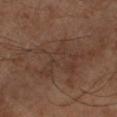Impression:
The lesion was photographed on a routine skin check and not biopsied; there is no pathology result.
Image and clinical context:
An algorithmic analysis of the crop reported an area of roughly 40 mm², an eccentricity of roughly 0.9, and a shape-asymmetry score of about 0.4 (0 = symmetric). The analysis additionally found an average lesion color of about L≈36 a*≈16 b*≈24 (CIELAB), about 5 CIELAB-L* units darker than the surrounding skin, and a lesion-to-skin contrast of about 5 (normalized; higher = more distinct). The analysis additionally found peripheral color asymmetry of about 1.5. And it measured a classifier nevus-likeness of about 0/100 and lesion-presence confidence of about 65/100. Located on the leg. A male subject, approximately 65 years of age. A roughly 15 mm field-of-view crop from a total-body skin photograph.